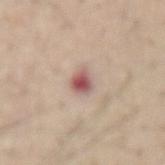Clinical impression:
The lesion was photographed on a routine skin check and not biopsied; there is no pathology result.
Background:
Captured under white-light illumination. An algorithmic analysis of the crop reported an automated nevus-likeness rating near 0 out of 100 and lesion-presence confidence of about 100/100. The lesion is located on the mid back. A male patient, aged 53–57. Cropped from a whole-body photographic skin survey; the tile spans about 15 mm.